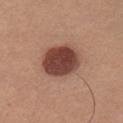workup: no biopsy performed (imaged during a skin exam)
size: ~5 mm (longest diameter)
lighting: white-light
image source: total-body-photography crop, ~15 mm field of view
automated metrics: an area of roughly 17 mm², an outline eccentricity of about 0.6 (0 = round, 1 = elongated), and two-axis asymmetry of about 0.1; a lesion color around L≈44 a*≈23 b*≈26 in CIELAB
body site: the chest
subject: male, about 35 years old